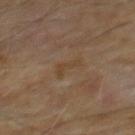Recorded during total-body skin imaging; not selected for excision or biopsy.